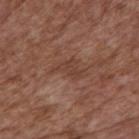Captured during whole-body skin photography for melanoma surveillance; the lesion was not biopsied. This image is a 15 mm lesion crop taken from a total-body photograph. A male patient, in their mid-70s. From the back.